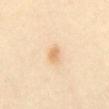| field | value |
|---|---|
| follow-up | no biopsy performed (imaged during a skin exam) |
| image | total-body-photography crop, ~15 mm field of view |
| image-analysis metrics | a lesion area of about 2.5 mm², an eccentricity of roughly 0.75, and a symmetry-axis asymmetry near 0.15; a color-variation rating of about 1.5/10 and peripheral color asymmetry of about 0.5; a classifier nevus-likeness of about 80/100 and lesion-presence confidence of about 100/100 |
| lesion diameter | ≈2 mm |
| anatomic site | the abdomen |
| tile lighting | cross-polarized illumination |
| subject | female, aged 48–52 |